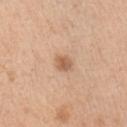| key | value |
|---|---|
| follow-up | imaged on a skin check; not biopsied |
| lesion size | about 2.5 mm |
| lighting | white-light |
| site | the right upper arm |
| acquisition | 15 mm crop, total-body photography |
| automated metrics | an area of roughly 3.5 mm², an eccentricity of roughly 0.65, and a shape-asymmetry score of about 0.2 (0 = symmetric) |
| patient | male, approximately 50 years of age |